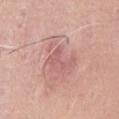| field | value |
|---|---|
| lesion size | ≈3.5 mm |
| image source | ~15 mm tile from a whole-body skin photo |
| tile lighting | white-light |
| anatomic site | the abdomen |
| subject | female, aged approximately 65 |